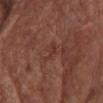Impression: The lesion was tiled from a total-body skin photograph and was not biopsied. Image and clinical context: Captured under white-light illumination. Measured at roughly 2.5 mm in maximum diameter. A 15 mm crop from a total-body photograph taken for skin-cancer surveillance. A female subject, aged approximately 80. The lesion is on the chest.